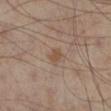Notes:
– biopsy status · imaged on a skin check; not biopsied
– diameter · ≈2 mm
– image-analysis metrics · a shape-asymmetry score of about 0.2 (0 = symmetric); an average lesion color of about L≈49 a*≈18 b*≈29 (CIELAB); a border-irregularity index near 1.5/10 and peripheral color asymmetry of about 0.5
– tile lighting · cross-polarized
– anatomic site · the left lower leg
– subject · male, about 55 years old
– image · ~15 mm crop, total-body skin-cancer survey